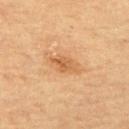This lesion was catalogued during total-body skin photography and was not selected for biopsy.
Automated tile analysis of the lesion measured roughly 9 lightness units darker than nearby skin and a normalized border contrast of about 6.5. The analysis additionally found a peripheral color-asymmetry measure near 1.5. And it measured a nevus-likeness score of about 10/100 and lesion-presence confidence of about 100/100.
A male patient aged approximately 65.
The lesion is on the upper back.
Captured under cross-polarized illumination.
This image is a 15 mm lesion crop taken from a total-body photograph.